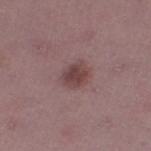Findings:
- workup — total-body-photography surveillance lesion; no biopsy
- location — the right thigh
- acquisition — ~15 mm crop, total-body skin-cancer survey
- lighting — white-light
- patient — female, in their mid- to late 40s